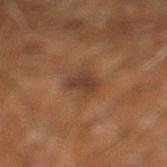Q: Was this lesion biopsied?
A: imaged on a skin check; not biopsied